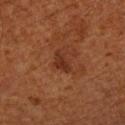biopsy_status: not biopsied; imaged during a skin examination
lesion_size:
  long_diameter_mm_approx: 3.0
patient:
  sex: male
  age_approx: 60
image:
  source: total-body photography crop
  field_of_view_mm: 15
lighting: cross-polarized
site: left upper arm